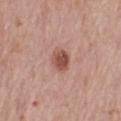No biopsy was performed on this lesion — it was imaged during a full skin examination and was not determined to be concerning.
This is a white-light tile.
A 15 mm crop from a total-body photograph taken for skin-cancer surveillance.
Automated tile analysis of the lesion measured a footprint of about 5 mm² and two-axis asymmetry of about 0.2. The analysis additionally found border irregularity of about 1.5 on a 0–10 scale. And it measured a detector confidence of about 100 out of 100 that the crop contains a lesion.
A female patient, aged 63–67.
The lesion's longest dimension is about 3 mm.
On the left thigh.